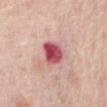The recorded lesion diameter is about 3 mm. Imaged with white-light lighting. The patient is a female about 65 years old. A 15 mm crop from a total-body photograph taken for skin-cancer surveillance. Located on the abdomen.